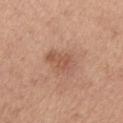This lesion was catalogued during total-body skin photography and was not selected for biopsy. Located on the arm. The patient is a female aged around 65. The recorded lesion diameter is about 4 mm. Cropped from a whole-body photographic skin survey; the tile spans about 15 mm.